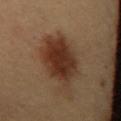notes = no biopsy performed (imaged during a skin exam) | image source = total-body-photography crop, ~15 mm field of view | lighting = cross-polarized illumination | lesion diameter = ≈8 mm | subject = female | location = the chest.